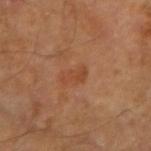Part of a total-body skin-imaging series; this lesion was reviewed on a skin check and was not flagged for biopsy.